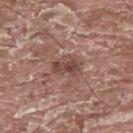Q: Is there a histopathology result?
A: imaged on a skin check; not biopsied
Q: What are the patient's age and sex?
A: male, aged approximately 40
Q: What is the imaging modality?
A: 15 mm crop, total-body photography
Q: Lesion size?
A: ≈3.5 mm
Q: Where on the body is the lesion?
A: the upper back
Q: Illumination type?
A: white-light